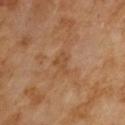follow-up — catalogued during a skin exam; not biopsied | lighting — cross-polarized | subject — male, in their mid- to late 60s | acquisition — 15 mm crop, total-body photography | size — ~3 mm (longest diameter).